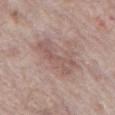follow-up: imaged on a skin check; not biopsied
lighting: white-light illumination
anatomic site: the abdomen
imaging modality: total-body-photography crop, ~15 mm field of view
automated lesion analysis: a footprint of about 13 mm², a shape eccentricity near 0.75, and two-axis asymmetry of about 0.4; a within-lesion color-variation index near 3.5/10 and peripheral color asymmetry of about 1.5
diameter: ≈5 mm
subject: male, roughly 70 years of age